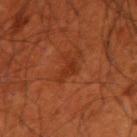No biopsy was performed on this lesion — it was imaged during a full skin examination and was not determined to be concerning. A region of skin cropped from a whole-body photographic capture, roughly 15 mm wide. A male patient aged 68–72. From the right upper arm.